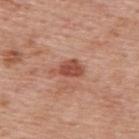{
  "biopsy_status": "not biopsied; imaged during a skin examination",
  "image": {
    "source": "total-body photography crop",
    "field_of_view_mm": 15
  },
  "patient": {
    "sex": "male",
    "age_approx": 75
  },
  "automated_metrics": {
    "area_mm2_approx": 6.0,
    "eccentricity": 0.85,
    "cielab_L": 50,
    "cielab_a": 27,
    "cielab_b": 31,
    "vs_skin_darker_L": 12.0,
    "vs_skin_contrast_norm": 8.5
  },
  "site": "upper back",
  "lesion_size": {
    "long_diameter_mm_approx": 4.0
  }
}